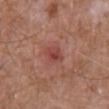Q: Is there a histopathology result?
A: catalogued during a skin exam; not biopsied
Q: Lesion location?
A: the mid back
Q: What kind of image is this?
A: total-body-photography crop, ~15 mm field of view
Q: How was the tile lit?
A: white-light
Q: How large is the lesion?
A: about 3 mm
Q: What did automated image analysis measure?
A: an average lesion color of about L≈45 a*≈28 b*≈25 (CIELAB), about 9 CIELAB-L* units darker than the surrounding skin, and a normalized border contrast of about 6.5
Q: Patient demographics?
A: male, approximately 60 years of age